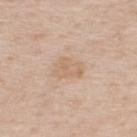Part of a total-body skin-imaging series; this lesion was reviewed on a skin check and was not flagged for biopsy. A male patient, in their 60s. A close-up tile cropped from a whole-body skin photograph, about 15 mm across. From the upper back.